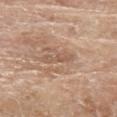| field | value |
|---|---|
| follow-up | imaged on a skin check; not biopsied |
| acquisition | ~15 mm crop, total-body skin-cancer survey |
| location | the right forearm |
| patient | female, aged approximately 75 |